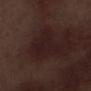Impression: Imaged during a routine full-body skin examination; the lesion was not biopsied and no histopathology is available. Acquisition and patient details: A male subject aged 68 to 72. From the leg. An algorithmic analysis of the crop reported a border-irregularity rating of about 5/10, a within-lesion color-variation index near 2/10, and peripheral color asymmetry of about 0.5. The recorded lesion diameter is about 4.5 mm. Cropped from a total-body skin-imaging series; the visible field is about 15 mm.